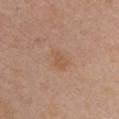This lesion was catalogued during total-body skin photography and was not selected for biopsy. A female patient, aged around 55. Cropped from a total-body skin-imaging series; the visible field is about 15 mm. Imaged with white-light lighting. The lesion is located on the chest. About 3 mm across.